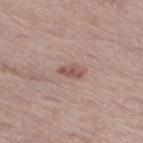  biopsy_status: not biopsied; imaged during a skin examination
  lesion_size:
    long_diameter_mm_approx: 3.0
  site: right thigh
  patient:
    sex: male
    age_approx: 65
  image:
    source: total-body photography crop
    field_of_view_mm: 15
  lighting: white-light
  automated_metrics:
    eccentricity: 0.85
    shape_asymmetry: 0.35
    vs_skin_darker_L: 10.0
    vs_skin_contrast_norm: 7.5
    nevus_likeness_0_100: 35
    lesion_detection_confidence_0_100: 100